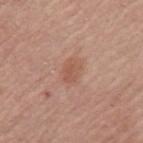• follow-up: total-body-photography surveillance lesion; no biopsy
• TBP lesion metrics: an eccentricity of roughly 0.8 and two-axis asymmetry of about 0.35; a lesion–skin lightness drop of about 8 and a normalized lesion–skin contrast near 5.5; peripheral color asymmetry of about 0.5; an automated nevus-likeness rating near 0 out of 100 and lesion-presence confidence of about 100/100
• image source: ~15 mm crop, total-body skin-cancer survey
• patient: female, aged approximately 65
• lesion diameter: about 2.5 mm
• site: the left upper arm
• lighting: white-light